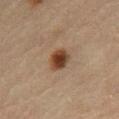The lesion was photographed on a routine skin check and not biopsied; there is no pathology result.
About 3 mm across.
A male patient aged approximately 70.
The tile uses cross-polarized illumination.
From the front of the torso.
A close-up tile cropped from a whole-body skin photograph, about 15 mm across.